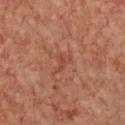{
  "biopsy_status": "not biopsied; imaged during a skin examination",
  "image": {
    "source": "total-body photography crop",
    "field_of_view_mm": 15
  },
  "patient": {
    "sex": "female",
    "age_approx": 45
  },
  "site": "chest",
  "lighting": "cross-polarized",
  "lesion_size": {
    "long_diameter_mm_approx": 3.0
  }
}